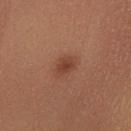Case summary:
– biopsy status — total-body-photography surveillance lesion; no biopsy
– illumination — cross-polarized illumination
– anatomic site — the right lower leg
– lesion size — about 2 mm
– patient — female, roughly 40 years of age
– imaging modality — total-body-photography crop, ~15 mm field of view